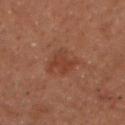Impression:
Captured during whole-body skin photography for melanoma surveillance; the lesion was not biopsied.
Image and clinical context:
A 15 mm close-up tile from a total-body photography series done for melanoma screening. Automated image analysis of the tile measured border irregularity of about 3.5 on a 0–10 scale, a color-variation rating of about 2/10, and a peripheral color-asymmetry measure near 1. The software also gave a nevus-likeness score of about 5/100 and lesion-presence confidence of about 100/100. Measured at roughly 3 mm in maximum diameter. The tile uses cross-polarized illumination. Located on the chest. A male patient, aged around 50.